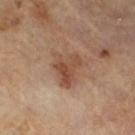Captured during whole-body skin photography for melanoma surveillance; the lesion was not biopsied. This is a cross-polarized tile. The patient is a male roughly 65 years of age. Approximately 4 mm at its widest. A 15 mm close-up extracted from a 3D total-body photography capture.